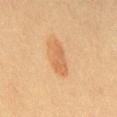Case summary:
– site · the back
– patient · female, aged 18 to 22
– acquisition · 15 mm crop, total-body photography